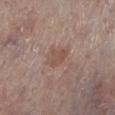Clinical impression: This lesion was catalogued during total-body skin photography and was not selected for biopsy. Image and clinical context: The tile uses white-light illumination. From the left lower leg. A lesion tile, about 15 mm wide, cut from a 3D total-body photograph. The lesion-visualizer software estimated a lesion color around L≈50 a*≈19 b*≈25 in CIELAB, a lesion–skin lightness drop of about 7, and a normalized lesion–skin contrast near 5.5. And it measured border irregularity of about 3.5 on a 0–10 scale, a color-variation rating of about 1.5/10, and a peripheral color-asymmetry measure near 0.5. The software also gave a classifier nevus-likeness of about 15/100 and a detector confidence of about 100 out of 100 that the crop contains a lesion. The lesion's longest dimension is about 2.5 mm. A male subject, aged approximately 80.